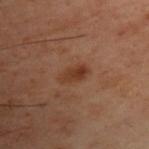notes: no biopsy performed (imaged during a skin exam).